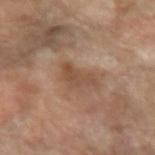Captured during whole-body skin photography for melanoma surveillance; the lesion was not biopsied.
A 15 mm crop from a total-body photograph taken for skin-cancer surveillance.
The subject is a female aged around 70.
Automated tile analysis of the lesion measured a lesion area of about 7 mm², an eccentricity of roughly 0.8, and a symmetry-axis asymmetry near 0.4. And it measured an average lesion color of about L≈50 a*≈18 b*≈30 (CIELAB). The software also gave border irregularity of about 4.5 on a 0–10 scale, internal color variation of about 2.5 on a 0–10 scale, and peripheral color asymmetry of about 0.5. It also reported a classifier nevus-likeness of about 0/100 and a detector confidence of about 100 out of 100 that the crop contains a lesion.
Located on the left forearm.